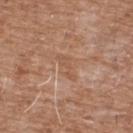Clinical impression: This lesion was catalogued during total-body skin photography and was not selected for biopsy. Clinical summary: A 15 mm crop from a total-body photograph taken for skin-cancer surveillance. The total-body-photography lesion software estimated a lesion area of about 3.5 mm², an eccentricity of roughly 0.95, and a symmetry-axis asymmetry near 0.45. And it measured a classifier nevus-likeness of about 0/100 and lesion-presence confidence of about 85/100. On the front of the torso. A male patient, aged 58 to 62. The recorded lesion diameter is about 3.5 mm.